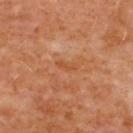No biopsy was performed on this lesion — it was imaged during a full skin examination and was not determined to be concerning. A 15 mm close-up tile from a total-body photography series done for melanoma screening. Approximately 2.5 mm at its widest. The patient is a female about 50 years old. The lesion is located on the back.